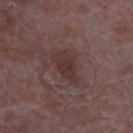Impression:
The lesion was tiled from a total-body skin photograph and was not biopsied.
Clinical summary:
Automated tile analysis of the lesion measured an area of roughly 6.5 mm², an outline eccentricity of about 0.85 (0 = round, 1 = elongated), and two-axis asymmetry of about 0.2. The analysis additionally found a lesion–skin lightness drop of about 7 and a lesion-to-skin contrast of about 6.5 (normalized; higher = more distinct). The analysis additionally found a border-irregularity index near 2.5/10, internal color variation of about 3 on a 0–10 scale, and radial color variation of about 1. It also reported a nevus-likeness score of about 0/100. On the chest. The lesion's longest dimension is about 4 mm. A 15 mm close-up tile from a total-body photography series done for melanoma screening. The subject is a male aged 63–67.